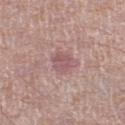Impression: The lesion was tiled from a total-body skin photograph and was not biopsied. Clinical summary: The lesion is on the left lower leg. The lesion's longest dimension is about 2.5 mm. A lesion tile, about 15 mm wide, cut from a 3D total-body photograph. The lesion-visualizer software estimated a classifier nevus-likeness of about 0/100 and a detector confidence of about 100 out of 100 that the crop contains a lesion. The tile uses white-light illumination. A male subject, aged 63–67.